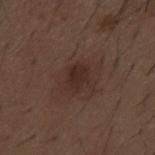Imaged during a routine full-body skin examination; the lesion was not biopsied and no histopathology is available.
A male subject, about 50 years old.
The lesion is on the back.
Imaged with white-light lighting.
The total-body-photography lesion software estimated an average lesion color of about L≈27 a*≈16 b*≈21 (CIELAB), roughly 6 lightness units darker than nearby skin, and a normalized lesion–skin contrast near 7. The software also gave a nevus-likeness score of about 10/100 and a lesion-detection confidence of about 100/100.
A 15 mm close-up tile from a total-body photography series done for melanoma screening.
The lesion's longest dimension is about 3 mm.